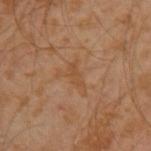Recorded during total-body skin imaging; not selected for excision or biopsy. The lesion is on the left upper arm. A male patient, aged around 30. Measured at roughly 2.5 mm in maximum diameter. A region of skin cropped from a whole-body photographic capture, roughly 15 mm wide.